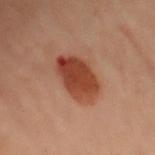<record>
  <biopsy_status>not biopsied; imaged during a skin examination</biopsy_status>
  <image>
    <source>total-body photography crop</source>
    <field_of_view_mm>15</field_of_view_mm>
  </image>
  <automated_metrics>
    <area_mm2_approx>15.0</area_mm2_approx>
    <eccentricity>0.85</eccentricity>
    <shape_asymmetry>0.15</shape_asymmetry>
    <cielab_L>37</cielab_L>
    <cielab_a>24</cielab_a>
    <cielab_b>29</cielab_b>
    <vs_skin_darker_L>12.0</vs_skin_darker_L>
    <vs_skin_contrast_norm>10.0</vs_skin_contrast_norm>
    <border_irregularity_0_10>2.0</border_irregularity_0_10>
    <color_variation_0_10>5.0</color_variation_0_10>
    <peripheral_color_asymmetry>2.0</peripheral_color_asymmetry>
  </automated_metrics>
  <lighting>cross-polarized</lighting>
  <lesion_size>
    <long_diameter_mm_approx>6.0</long_diameter_mm_approx>
  </lesion_size>
  <patient>
    <sex>female</sex>
    <age_approx>60</age_approx>
  </patient>
  <site>right arm</site>
</record>